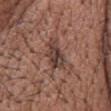notes: imaged on a skin check; not biopsied
subject: male, about 65 years old
lighting: white-light
site: the head or neck
image: ~15 mm crop, total-body skin-cancer survey